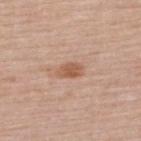No biopsy was performed on this lesion — it was imaged during a full skin examination and was not determined to be concerning. The lesion-visualizer software estimated a mean CIELAB color near L≈57 a*≈21 b*≈31, a lesion–skin lightness drop of about 10, and a normalized lesion–skin contrast near 7.5. And it measured a classifier nevus-likeness of about 85/100. On the upper back. Cropped from a whole-body photographic skin survey; the tile spans about 15 mm. A female subject roughly 50 years of age. The lesion's longest dimension is about 3 mm. Captured under white-light illumination.